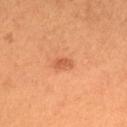Recorded during total-body skin imaging; not selected for excision or biopsy.
The lesion is located on the head or neck.
A female subject aged 28–32.
A 15 mm close-up extracted from a 3D total-body photography capture.
Imaged with cross-polarized lighting.